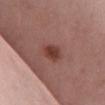workup: total-body-photography surveillance lesion; no biopsy
acquisition: total-body-photography crop, ~15 mm field of view
site: the chest
automated metrics: an automated nevus-likeness rating near 95 out of 100 and a detector confidence of about 100 out of 100 that the crop contains a lesion
tile lighting: white-light illumination
patient: female, roughly 40 years of age